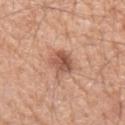Image and clinical context:
The lesion is on the left forearm. A 15 mm close-up tile from a total-body photography series done for melanoma screening. A male patient approximately 50 years of age.